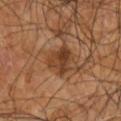Imaged during a routine full-body skin examination; the lesion was not biopsied and no histopathology is available. An algorithmic analysis of the crop reported a mean CIELAB color near L≈37 a*≈21 b*≈31 and roughly 10 lightness units darker than nearby skin. It also reported border irregularity of about 4.5 on a 0–10 scale, internal color variation of about 4.5 on a 0–10 scale, and peripheral color asymmetry of about 1.5. A male subject aged 58 to 62. The lesion is located on the right arm. About 4 mm across. A 15 mm close-up extracted from a 3D total-body photography capture. The tile uses cross-polarized illumination.